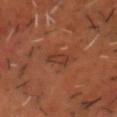follow-up: catalogued during a skin exam; not biopsied | illumination: cross-polarized illumination | subject: male, aged around 50 | acquisition: 15 mm crop, total-body photography | site: the head or neck.